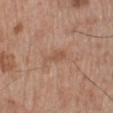biopsy_status: not biopsied; imaged during a skin examination
image:
  source: total-body photography crop
  field_of_view_mm: 15
patient:
  sex: male
  age_approx: 70
site: left lower leg
automated_metrics:
  area_mm2_approx: 3.0
  eccentricity: 0.9
  shape_asymmetry: 0.35
  color_variation_0_10: 0.5
  peripheral_color_asymmetry: 0.5
lighting: white-light